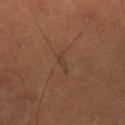Q: Is there a histopathology result?
A: catalogued during a skin exam; not biopsied
Q: What is the imaging modality?
A: 15 mm crop, total-body photography
Q: How large is the lesion?
A: about 2.5 mm
Q: Illumination type?
A: cross-polarized illumination
Q: Patient demographics?
A: male, in their 50s
Q: Automated lesion metrics?
A: an average lesion color of about L≈37 a*≈17 b*≈27 (CIELAB), a lesion–skin lightness drop of about 5, and a lesion-to-skin contrast of about 4.5 (normalized; higher = more distinct); a border-irregularity index near 4.5/10, internal color variation of about 0 on a 0–10 scale, and peripheral color asymmetry of about 0
Q: Where on the body is the lesion?
A: the right lower leg